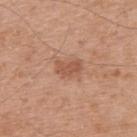Case summary:
• biopsy status: total-body-photography surveillance lesion; no biopsy
• patient: male, about 55 years old
• body site: the upper back
• image: ~15 mm crop, total-body skin-cancer survey